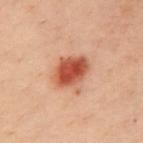biopsy_status: not biopsied; imaged during a skin examination
patient:
  sex: male
  age_approx: 40
lesion_size:
  long_diameter_mm_approx: 4.5
site: chest
lighting: cross-polarized
automated_metrics:
  area_mm2_approx: 12.0
  shape_asymmetry: 0.15
  cielab_L: 43
  cielab_a: 25
  cielab_b: 28
  vs_skin_darker_L: 13.0
  vs_skin_contrast_norm: 10.0
  border_irregularity_0_10: 1.5
  peripheral_color_asymmetry: 1.5
image:
  source: total-body photography crop
  field_of_view_mm: 15